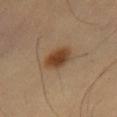<record>
<site>chest</site>
<patient>
  <sex>male</sex>
  <age_approx>55</age_approx>
</patient>
<image>
  <source>total-body photography crop</source>
  <field_of_view_mm>15</field_of_view_mm>
</image>
</record>